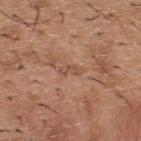No biopsy was performed on this lesion — it was imaged during a full skin examination and was not determined to be concerning. A close-up tile cropped from a whole-body skin photograph, about 15 mm across. The lesion is on the back. A male patient, roughly 40 years of age.